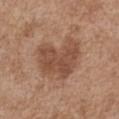No biopsy was performed on this lesion — it was imaged during a full skin examination and was not determined to be concerning.
Automated tile analysis of the lesion measured a peripheral color-asymmetry measure near 1. The software also gave a nevus-likeness score of about 50/100.
The patient is a female aged 73–77.
Located on the chest.
The tile uses white-light illumination.
This image is a 15 mm lesion crop taken from a total-body photograph.